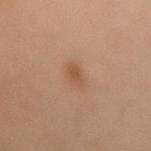Clinical impression:
Recorded during total-body skin imaging; not selected for excision or biopsy.
Background:
From the lower back. The tile uses cross-polarized illumination. The subject is a male approximately 60 years of age. About 3 mm across. A 15 mm close-up tile from a total-body photography series done for melanoma screening.